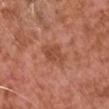– workup — total-body-photography surveillance lesion; no biopsy
– site — the chest
– subject — male, aged approximately 75
– image source — total-body-photography crop, ~15 mm field of view
– lesion diameter — ~4 mm (longest diameter)
– illumination — white-light illumination